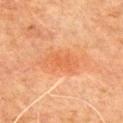Clinical impression:
The lesion was photographed on a routine skin check and not biopsied; there is no pathology result.
Image and clinical context:
Imaged with cross-polarized lighting. A roughly 15 mm field-of-view crop from a total-body skin photograph. From the front of the torso. A male patient, aged 78 to 82.